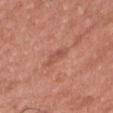Clinical impression: Captured during whole-body skin photography for melanoma surveillance; the lesion was not biopsied. Image and clinical context: The lesion's longest dimension is about 2.5 mm. A male subject aged 23 to 27. A 15 mm close-up tile from a total-body photography series done for melanoma screening. The lesion is located on the head or neck. Imaged with white-light lighting.